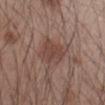On the arm.
A 15 mm close-up tile from a total-body photography series done for melanoma screening.
Imaged with white-light lighting.
The recorded lesion diameter is about 3.5 mm.
A male patient, approximately 45 years of age.